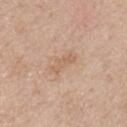Clinical impression: No biopsy was performed on this lesion — it was imaged during a full skin examination and was not determined to be concerning. Acquisition and patient details: The total-body-photography lesion software estimated an outline eccentricity of about 0.9 (0 = round, 1 = elongated) and a symmetry-axis asymmetry near 0.5. It also reported a border-irregularity rating of about 6/10 and peripheral color asymmetry of about 0. And it measured a classifier nevus-likeness of about 0/100. A male patient, in their mid- to late 50s. From the chest. A 15 mm close-up extracted from a 3D total-body photography capture. Imaged with white-light lighting. The lesion's longest dimension is about 3 mm.